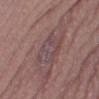notes: total-body-photography surveillance lesion; no biopsy | illumination: white-light illumination | image: 15 mm crop, total-body photography | lesion size: ~6.5 mm (longest diameter) | subject: female, aged around 40 | TBP lesion metrics: a lesion color around L≈46 a*≈17 b*≈15 in CIELAB and roughly 6 lightness units darker than nearby skin; a nevus-likeness score of about 0/100 and a lesion-detection confidence of about 10/100 | body site: the right thigh.